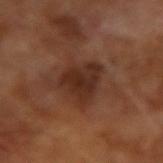| field | value |
|---|---|
| illumination | cross-polarized illumination |
| acquisition | ~15 mm crop, total-body skin-cancer survey |
| patient | male, approximately 65 years of age |
| diameter | ~4.5 mm (longest diameter) |